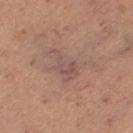| field | value |
|---|---|
| biopsy status | imaged on a skin check; not biopsied |
| tile lighting | white-light |
| imaging modality | 15 mm crop, total-body photography |
| body site | the left thigh |
| subject | female, aged approximately 40 |
| TBP lesion metrics | a nevus-likeness score of about 0/100 |